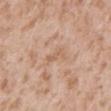Imaged during a routine full-body skin examination; the lesion was not biopsied and no histopathology is available. A male subject, in their mid- to late 40s. A region of skin cropped from a whole-body photographic capture, roughly 15 mm wide. The lesion is located on the back.